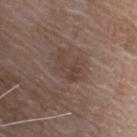follow-up: imaged on a skin check; not biopsied
body site: the upper back
lesion diameter: ≈3.5 mm
acquisition: ~15 mm tile from a whole-body skin photo
subject: female, aged 73 to 77